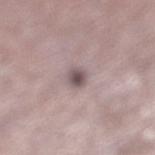A male patient, about 65 years old. On the right lower leg. A lesion tile, about 15 mm wide, cut from a 3D total-body photograph.